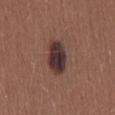The lesion was photographed on a routine skin check and not biopsied; there is no pathology result.
Imaged with white-light lighting.
This image is a 15 mm lesion crop taken from a total-body photograph.
From the mid back.
A female subject approximately 25 years of age.